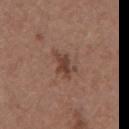- follow-up: no biopsy performed (imaged during a skin exam)
- lighting: white-light
- image source: ~15 mm tile from a whole-body skin photo
- lesion diameter: about 4 mm
- automated lesion analysis: an average lesion color of about L≈41 a*≈20 b*≈26 (CIELAB), about 10 CIELAB-L* units darker than the surrounding skin, and a normalized lesion–skin contrast near 8; a color-variation rating of about 2/10; a nevus-likeness score of about 5/100 and a lesion-detection confidence of about 100/100
- subject: female, approximately 65 years of age
- location: the chest